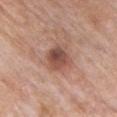Notes:
- biopsy status · imaged on a skin check; not biopsied
- site · the chest
- illumination · white-light
- size · ≈3.5 mm
- subject · female, roughly 60 years of age
- image source · ~15 mm tile from a whole-body skin photo